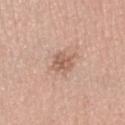Measured at roughly 2.5 mm in maximum diameter. Automated tile analysis of the lesion measured a mean CIELAB color near L≈59 a*≈20 b*≈29, roughly 10 lightness units darker than nearby skin, and a normalized lesion–skin contrast near 6.5. And it measured a border-irregularity index near 3/10, a color-variation rating of about 3/10, and a peripheral color-asymmetry measure near 1. And it measured an automated nevus-likeness rating near 5 out of 100 and a lesion-detection confidence of about 100/100. This is a white-light tile. The patient is a male aged approximately 25. The lesion is on the head or neck. A 15 mm close-up tile from a total-body photography series done for melanoma screening.